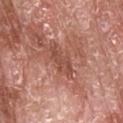| key | value |
|---|---|
| image | ~15 mm tile from a whole-body skin photo |
| lesion size | ~4.5 mm (longest diameter) |
| patient | male, aged 63–67 |
| site | the head or neck |